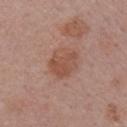biopsy_status: not biopsied; imaged during a skin examination
image:
  source: total-body photography crop
  field_of_view_mm: 15
patient:
  sex: female
  age_approx: 55
site: right upper arm